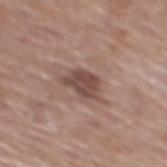Case summary:
* workup: imaged on a skin check; not biopsied
* lesion size: ≈4 mm
* patient: male, in their mid-70s
* body site: the mid back
* acquisition: ~15 mm tile from a whole-body skin photo
* tile lighting: white-light